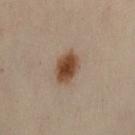workup: catalogued during a skin exam; not biopsied
location: the abdomen
size: about 3.5 mm
image source: 15 mm crop, total-body photography
subject: female, in their 50s